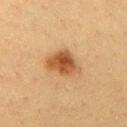Q: How was this image acquired?
A: ~15 mm crop, total-body skin-cancer survey
Q: What is the anatomic site?
A: the chest
Q: Illumination type?
A: cross-polarized illumination
Q: Patient demographics?
A: female, aged 38 to 42